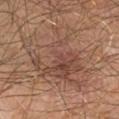- notes — catalogued during a skin exam; not biopsied
- body site — the right upper arm
- diameter — ~9.5 mm (longest diameter)
- lighting — cross-polarized
- acquisition — 15 mm crop, total-body photography
- patient — male, roughly 65 years of age